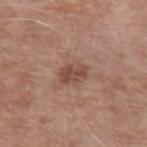Imaged during a routine full-body skin examination; the lesion was not biopsied and no histopathology is available.
Captured under white-light illumination.
From the arm.
Measured at roughly 3.5 mm in maximum diameter.
Cropped from a whole-body photographic skin survey; the tile spans about 15 mm.
The patient is a male aged 68–72.